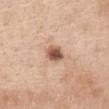Imaged during a routine full-body skin examination; the lesion was not biopsied and no histopathology is available. A close-up tile cropped from a whole-body skin photograph, about 15 mm across. The lesion's longest dimension is about 3 mm. The patient is a female about 40 years old. The lesion-visualizer software estimated an outline eccentricity of about 0.65 (0 = round, 1 = elongated). It also reported an automated nevus-likeness rating near 95 out of 100 and a detector confidence of about 100 out of 100 that the crop contains a lesion. This is a white-light tile. On the front of the torso.